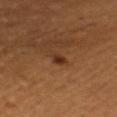<tbp_lesion>
  <lesion_size>
    <long_diameter_mm_approx>2.5</long_diameter_mm_approx>
  </lesion_size>
  <site>right upper arm</site>
  <image>
    <source>total-body photography crop</source>
    <field_of_view_mm>15</field_of_view_mm>
  </image>
  <patient>
    <sex>female</sex>
    <age_approx>30</age_approx>
  </patient>
</tbp_lesion>